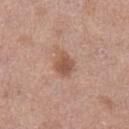notes = imaged on a skin check; not biopsied
body site = the left thigh
lighting = white-light
imaging modality = ~15 mm crop, total-body skin-cancer survey
automated metrics = a mean CIELAB color near L≈53 a*≈21 b*≈29, roughly 10 lightness units darker than nearby skin, and a normalized border contrast of about 7.5; an automated nevus-likeness rating near 60 out of 100 and a detector confidence of about 100 out of 100 that the crop contains a lesion
patient = female, aged 38 to 42